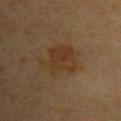This lesion was catalogued during total-body skin photography and was not selected for biopsy. A female patient about 55 years old. This is a cross-polarized tile. The total-body-photography lesion software estimated a lesion–skin lightness drop of about 5 and a normalized border contrast of about 7. A 15 mm close-up tile from a total-body photography series done for melanoma screening. From the upper back. The lesion's longest dimension is about 5 mm.